A 15 mm close-up extracted from a 3D total-body photography capture · the subject is a female roughly 55 years of age · on the chest: 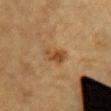Histopathologically confirmed as an intradermal melanocytic nevus.